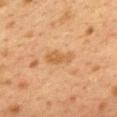No biopsy was performed on this lesion — it was imaged during a full skin examination and was not determined to be concerning. The tile uses cross-polarized illumination. This image is a 15 mm lesion crop taken from a total-body photograph. The patient is a female in their 40s. On the upper back. Automated tile analysis of the lesion measured a lesion area of about 5.5 mm² and a shape-asymmetry score of about 0.25 (0 = symmetric). It also reported a border-irregularity index near 3/10, internal color variation of about 2 on a 0–10 scale, and a peripheral color-asymmetry measure near 0.5. It also reported a nevus-likeness score of about 25/100 and a detector confidence of about 100 out of 100 that the crop contains a lesion.